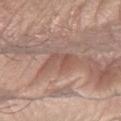Case summary:
- notes: catalogued during a skin exam; not biopsied
- patient: male, aged 53 to 57
- image source: total-body-photography crop, ~15 mm field of view
- anatomic site: the arm